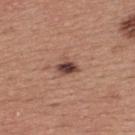Clinical summary: A region of skin cropped from a whole-body photographic capture, roughly 15 mm wide. The subject is a male aged 38 to 42. Captured under white-light illumination. On the upper back. Automated tile analysis of the lesion measured a footprint of about 4 mm² and a shape eccentricity near 0.7. It also reported a classifier nevus-likeness of about 95/100 and a lesion-detection confidence of about 100/100.